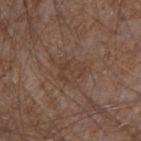notes — imaged on a skin check; not biopsied
lesion size — about 4.5 mm
site — the right forearm
patient — male, about 75 years old
lighting — white-light illumination
TBP lesion metrics — an eccentricity of roughly 0.6; a mean CIELAB color near L≈40 a*≈16 b*≈25, roughly 5 lightness units darker than nearby skin, and a normalized lesion–skin contrast near 5; a border-irregularity index near 5/10 and a within-lesion color-variation index near 2.5/10
image source — total-body-photography crop, ~15 mm field of view